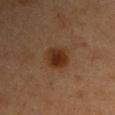Part of a total-body skin-imaging series; this lesion was reviewed on a skin check and was not flagged for biopsy.